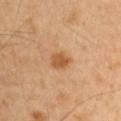| field | value |
|---|---|
| anatomic site | the chest |
| automated metrics | a footprint of about 4.5 mm², a shape eccentricity near 0.55, and two-axis asymmetry of about 0.25; roughly 10 lightness units darker than nearby skin and a normalized lesion–skin contrast near 8; a nevus-likeness score of about 90/100 and a detector confidence of about 100 out of 100 that the crop contains a lesion |
| patient | male, roughly 60 years of age |
| illumination | cross-polarized illumination |
| diameter | ≈2.5 mm |
| image source | ~15 mm tile from a whole-body skin photo |